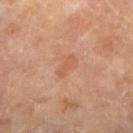biopsy status = catalogued during a skin exam; not biopsied | lesion diameter = ~2.5 mm (longest diameter) | subject = female, roughly 70 years of age | acquisition = ~15 mm crop, total-body skin-cancer survey | illumination = cross-polarized | body site = the left lower leg.